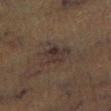Clinical impression: The lesion was tiled from a total-body skin photograph and was not biopsied. Acquisition and patient details: Automated image analysis of the tile measured a lesion area of about 8.5 mm² and two-axis asymmetry of about 0.35. And it measured border irregularity of about 3.5 on a 0–10 scale, internal color variation of about 4.5 on a 0–10 scale, and peripheral color asymmetry of about 1.5. The analysis additionally found a lesion-detection confidence of about 80/100. The patient is a male roughly 75 years of age. Located on the right lower leg. Captured under cross-polarized illumination. About 4 mm across. A 15 mm close-up tile from a total-body photography series done for melanoma screening.